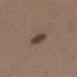The lesion was photographed on a routine skin check and not biopsied; there is no pathology result.
The subject is a female approximately 40 years of age.
About 3 mm across.
This is a white-light tile.
A 15 mm close-up extracted from a 3D total-body photography capture.
Automated image analysis of the tile measured a footprint of about 5 mm² and a shape-asymmetry score of about 0.15 (0 = symmetric). The analysis additionally found a border-irregularity index near 1.5/10 and a color-variation rating of about 3.5/10.
The lesion is on the leg.